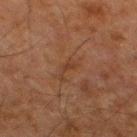Q: Was this lesion biopsied?
A: catalogued during a skin exam; not biopsied
Q: How was the tile lit?
A: cross-polarized illumination
Q: How large is the lesion?
A: ≈3.5 mm
Q: Who is the patient?
A: male, aged 78–82
Q: Automated lesion metrics?
A: an outline eccentricity of about 0.85 (0 = round, 1 = elongated); an average lesion color of about L≈30 a*≈17 b*≈25 (CIELAB), roughly 4 lightness units darker than nearby skin, and a lesion-to-skin contrast of about 5 (normalized; higher = more distinct); an automated nevus-likeness rating near 0 out of 100 and lesion-presence confidence of about 100/100
Q: Where on the body is the lesion?
A: the right thigh
Q: What is the imaging modality?
A: ~15 mm crop, total-body skin-cancer survey